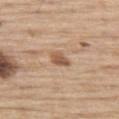The lesion is on the leg. The recorded lesion diameter is about 2.5 mm. A male subject about 70 years old. Captured under white-light illumination. An algorithmic analysis of the crop reported a mean CIELAB color near L≈56 a*≈18 b*≈32, roughly 11 lightness units darker than nearby skin, and a lesion-to-skin contrast of about 7.5 (normalized; higher = more distinct). And it measured a nevus-likeness score of about 60/100 and a lesion-detection confidence of about 100/100. Cropped from a total-body skin-imaging series; the visible field is about 15 mm.